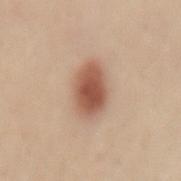Captured during whole-body skin photography for melanoma surveillance; the lesion was not biopsied. An algorithmic analysis of the crop reported a footprint of about 12 mm², an outline eccentricity of about 0.8 (0 = round, 1 = elongated), and a shape-asymmetry score of about 0.15 (0 = symmetric). The software also gave roughly 15 lightness units darker than nearby skin and a normalized lesion–skin contrast near 10. And it measured a nevus-likeness score of about 100/100 and a lesion-detection confidence of about 100/100. A 15 mm close-up tile from a total-body photography series done for melanoma screening. A female subject, in their 50s. Measured at roughly 5 mm in maximum diameter. From the lower back.